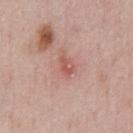subject: male, approximately 50 years of age
imaging modality: total-body-photography crop, ~15 mm field of view
location: the abdomen